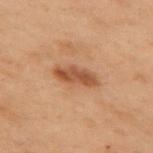Clinical impression:
The lesion was tiled from a total-body skin photograph and was not biopsied.
Image and clinical context:
A roughly 15 mm field-of-view crop from a total-body skin photograph. The patient is a female in their mid-50s. From the left arm.